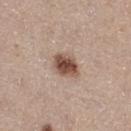Notes:
– workup · total-body-photography surveillance lesion; no biopsy
– tile lighting · white-light
– patient · female, about 50 years old
– lesion size · ≈3.5 mm
– automated lesion analysis · a footprint of about 7 mm², an outline eccentricity of about 0.55 (0 = round, 1 = elongated), and a shape-asymmetry score of about 0.15 (0 = symmetric); roughly 16 lightness units darker than nearby skin and a normalized border contrast of about 11; border irregularity of about 1.5 on a 0–10 scale, a color-variation rating of about 4.5/10, and radial color variation of about 1.5
– image · total-body-photography crop, ~15 mm field of view
– anatomic site · the right thigh